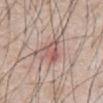* workup — no biopsy performed (imaged during a skin exam)
* imaging modality — total-body-photography crop, ~15 mm field of view
* location — the chest
* lesion size — ≈4 mm
* subject — male, roughly 65 years of age